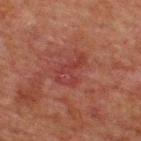Q: Was a biopsy performed?
A: no biopsy performed (imaged during a skin exam)
Q: What is the anatomic site?
A: the upper back
Q: What are the patient's age and sex?
A: male, approximately 60 years of age
Q: How was this image acquired?
A: total-body-photography crop, ~15 mm field of view